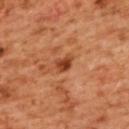Assessment: Imaged during a routine full-body skin examination; the lesion was not biopsied and no histopathology is available. Image and clinical context: The recorded lesion diameter is about 2.5 mm. A region of skin cropped from a whole-body photographic capture, roughly 15 mm wide. From the back. A female patient in their mid- to late 50s. The tile uses cross-polarized illumination.